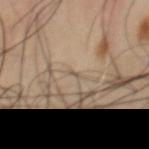Case summary:
- lighting: cross-polarized
- diameter: about 1.5 mm
- patient: male, aged 53 to 57
- image: ~15 mm crop, total-body skin-cancer survey
- site: the chest